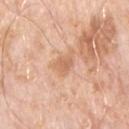No biopsy was performed on this lesion — it was imaged during a full skin examination and was not determined to be concerning. A close-up tile cropped from a whole-body skin photograph, about 15 mm across. The subject is a male roughly 70 years of age. The recorded lesion diameter is about 2.5 mm. The lesion is on the chest. Captured under white-light illumination.